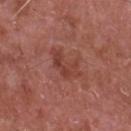Q: Is there a histopathology result?
A: total-body-photography surveillance lesion; no biopsy
Q: What is the anatomic site?
A: the chest
Q: What kind of image is this?
A: ~15 mm tile from a whole-body skin photo
Q: What are the patient's age and sex?
A: male, aged 63–67